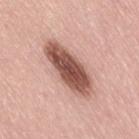Findings:
– notes · catalogued during a skin exam; not biopsied
– lesion size · ~8 mm (longest diameter)
– automated lesion analysis · a lesion color around L≈54 a*≈23 b*≈26 in CIELAB, a lesion–skin lightness drop of about 19, and a normalized border contrast of about 11.5; border irregularity of about 3 on a 0–10 scale, internal color variation of about 5.5 on a 0–10 scale, and a peripheral color-asymmetry measure near 2
– patient · female, approximately 65 years of age
– site · the right thigh
– tile lighting · white-light illumination
– imaging modality · ~15 mm crop, total-body skin-cancer survey